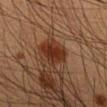Imaged during a routine full-body skin examination; the lesion was not biopsied and no histopathology is available.